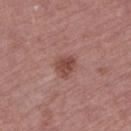A region of skin cropped from a whole-body photographic capture, roughly 15 mm wide.
The patient is a female in their mid-70s.
The lesion is located on the left lower leg.
The total-body-photography lesion software estimated an outline eccentricity of about 0.5 (0 = round, 1 = elongated) and a shape-asymmetry score of about 0.3 (0 = symmetric). The analysis additionally found an average lesion color of about L≈46 a*≈24 b*≈24 (CIELAB), roughly 10 lightness units darker than nearby skin, and a lesion-to-skin contrast of about 7.5 (normalized; higher = more distinct). And it measured internal color variation of about 2.5 on a 0–10 scale and peripheral color asymmetry of about 1. The analysis additionally found a nevus-likeness score of about 75/100 and a lesion-detection confidence of about 100/100.